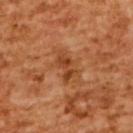Q: Was a biopsy performed?
A: no biopsy performed (imaged during a skin exam)
Q: Patient demographics?
A: female, roughly 55 years of age
Q: What is the anatomic site?
A: the upper back
Q: What is the imaging modality?
A: total-body-photography crop, ~15 mm field of view